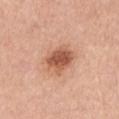- workup — no biopsy performed (imaged during a skin exam)
- location — the arm
- patient — male, aged 73 to 77
- image source — 15 mm crop, total-body photography